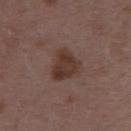Notes:
* notes — imaged on a skin check; not biopsied
* illumination — white-light
* subject — female, in their mid- to late 40s
* location — the upper back
* image — 15 mm crop, total-body photography
* diameter — about 4 mm